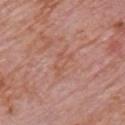Impression: Recorded during total-body skin imaging; not selected for excision or biopsy. Context: A male patient roughly 80 years of age. The lesion-visualizer software estimated a border-irregularity index near 5/10 and a peripheral color-asymmetry measure near 0.5. About 3 mm across. The lesion is on the chest. A lesion tile, about 15 mm wide, cut from a 3D total-body photograph.